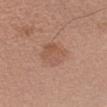{"lesion_size": {"long_diameter_mm_approx": 4.0}, "site": "left forearm", "image": {"source": "total-body photography crop", "field_of_view_mm": 15}, "automated_metrics": {"eccentricity": 0.6, "cielab_L": 54, "cielab_a": 21, "cielab_b": 30, "vs_skin_darker_L": 7.0}, "patient": {"sex": "female", "age_approx": 35}, "lighting": "white-light"}